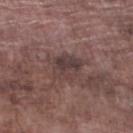{"biopsy_status": "not biopsied; imaged during a skin examination", "lesion_size": {"long_diameter_mm_approx": 3.5}, "lighting": "white-light", "site": "left lower leg", "automated_metrics": {"cielab_L": 38, "cielab_a": 16, "cielab_b": 16, "border_irregularity_0_10": 3.0, "color_variation_0_10": 3.0, "peripheral_color_asymmetry": 1.0, "nevus_likeness_0_100": 0, "lesion_detection_confidence_0_100": 95}, "patient": {"sex": "male", "age_approx": 75}, "image": {"source": "total-body photography crop", "field_of_view_mm": 15}}